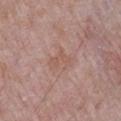Imaged during a routine full-body skin examination; the lesion was not biopsied and no histopathology is available. On the arm. The subject is a male aged around 65. Measured at roughly 3 mm in maximum diameter. Automated tile analysis of the lesion measured an area of roughly 4.5 mm² and an eccentricity of roughly 0.75. And it measured a normalized border contrast of about 4.5. The analysis additionally found a border-irregularity index near 4.5/10 and peripheral color asymmetry of about 0.5. The software also gave a nevus-likeness score of about 0/100. Cropped from a total-body skin-imaging series; the visible field is about 15 mm.